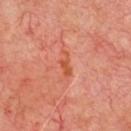Assessment: Recorded during total-body skin imaging; not selected for excision or biopsy. Clinical summary: About 3.5 mm across. From the chest. The patient is a male in their mid- to late 60s. A 15 mm close-up tile from a total-body photography series done for melanoma screening. This is a cross-polarized tile.